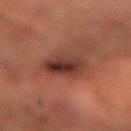{"automated_metrics": {"area_mm2_approx": 12.0, "eccentricity": 0.65, "shape_asymmetry": 0.15, "color_variation_0_10": 8.0}, "image": {"source": "total-body photography crop", "field_of_view_mm": 15}, "lighting": "cross-polarized", "site": "left forearm", "patient": {"sex": "male", "age_approx": 65}}